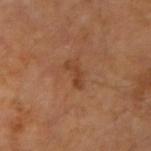{"biopsy_status": "not biopsied; imaged during a skin examination", "image": {"source": "total-body photography crop", "field_of_view_mm": 15}, "lighting": "cross-polarized", "automated_metrics": {"area_mm2_approx": 2.5, "shape_asymmetry": 0.6, "cielab_L": 40, "cielab_a": 24, "cielab_b": 33, "vs_skin_darker_L": 8.0, "vs_skin_contrast_norm": 6.5, "nevus_likeness_0_100": 0, "lesion_detection_confidence_0_100": 100}, "site": "right upper arm", "patient": {"sex": "male", "age_approx": 70}, "lesion_size": {"long_diameter_mm_approx": 3.0}}